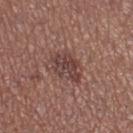{
  "biopsy_status": "not biopsied; imaged during a skin examination",
  "image": {
    "source": "total-body photography crop",
    "field_of_view_mm": 15
  },
  "site": "right thigh",
  "automated_metrics": {
    "area_mm2_approx": 8.0,
    "eccentricity": 0.7,
    "shape_asymmetry": 0.35
  },
  "patient": {
    "sex": "female",
    "age_approx": 25
  },
  "lighting": "white-light"
}